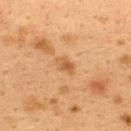Case summary:
- biopsy status: catalogued during a skin exam; not biopsied
- imaging modality: ~15 mm tile from a whole-body skin photo
- patient: female, aged 38–42
- body site: the upper back